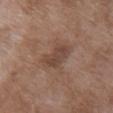* biopsy status: imaged on a skin check; not biopsied
* size: ~4 mm (longest diameter)
* site: the upper back
* tile lighting: white-light
* image source: 15 mm crop, total-body photography
* patient: female, in their 70s